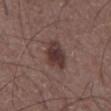<tbp_lesion>
<biopsy_status>not biopsied; imaged during a skin examination</biopsy_status>
<image>
  <source>total-body photography crop</source>
  <field_of_view_mm>15</field_of_view_mm>
</image>
<automated_metrics>
  <cielab_L>35</cielab_L>
  <cielab_a>17</cielab_a>
  <cielab_b>19</cielab_b>
  <vs_skin_darker_L>10.0</vs_skin_darker_L>
</automated_metrics>
<site>abdomen</site>
<lesion_size>
  <long_diameter_mm_approx>4.0</long_diameter_mm_approx>
</lesion_size>
<patient>
  <sex>male</sex>
  <age_approx>60</age_approx>
</patient>
</tbp_lesion>